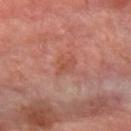<lesion>
<biopsy_status>not biopsied; imaged during a skin examination</biopsy_status>
<site>left forearm</site>
<patient>
  <sex>female</sex>
  <age_approx>65</age_approx>
</patient>
<image>
  <source>total-body photography crop</source>
  <field_of_view_mm>15</field_of_view_mm>
</image>
</lesion>